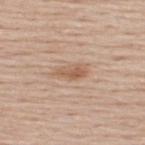workup: total-body-photography surveillance lesion; no biopsy
patient: male, in their 60s
image source: ~15 mm tile from a whole-body skin photo
body site: the upper back